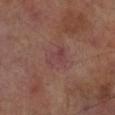<lesion>
<biopsy_status>not biopsied; imaged during a skin examination</biopsy_status>
<patient>
  <sex>male</sex>
  <age_approx>70</age_approx>
</patient>
<automated_metrics>
  <area_mm2_approx>4.5</area_mm2_approx>
  <eccentricity>0.7</eccentricity>
  <border_irregularity_0_10>6.0</border_irregularity_0_10>
  <color_variation_0_10>2.0</color_variation_0_10>
  <peripheral_color_asymmetry>0.5</peripheral_color_asymmetry>
  <nevus_likeness_0_100>0</nevus_likeness_0_100>
</automated_metrics>
<lesion_size>
  <long_diameter_mm_approx>3.0</long_diameter_mm_approx>
</lesion_size>
<lighting>cross-polarized</lighting>
<site>right lower leg</site>
<image>
  <source>total-body photography crop</source>
  <field_of_view_mm>15</field_of_view_mm>
</image>
</lesion>